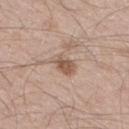Q: Was a biopsy performed?
A: no biopsy performed (imaged during a skin exam)
Q: What is the imaging modality?
A: 15 mm crop, total-body photography
Q: What are the patient's age and sex?
A: male, approximately 65 years of age
Q: Illumination type?
A: white-light
Q: What is the lesion's diameter?
A: ≈3 mm
Q: Where on the body is the lesion?
A: the right thigh
Q: What did automated image analysis measure?
A: an average lesion color of about L≈55 a*≈17 b*≈27 (CIELAB), a lesion–skin lightness drop of about 11, and a lesion-to-skin contrast of about 7.5 (normalized; higher = more distinct); a border-irregularity index near 2.5/10 and a within-lesion color-variation index near 3/10; a nevus-likeness score of about 60/100 and a detector confidence of about 100 out of 100 that the crop contains a lesion